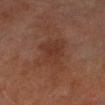Cropped from a whole-body photographic skin survey; the tile spans about 15 mm. A male patient, aged 68 to 72. This is a cross-polarized tile. The lesion is located on the left lower leg.